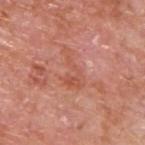| feature | finding |
|---|---|
| notes | no biopsy performed (imaged during a skin exam) |
| imaging modality | ~15 mm tile from a whole-body skin photo |
| patient | male, aged 63 to 67 |
| anatomic site | the upper back |